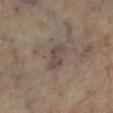notes = imaged on a skin check; not biopsied | imaging modality = total-body-photography crop, ~15 mm field of view | tile lighting = cross-polarized | patient = male, about 70 years old | site = the right lower leg.